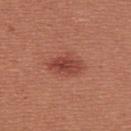The lesion was tiled from a total-body skin photograph and was not biopsied. The lesion-visualizer software estimated an area of roughly 7.5 mm² and a shape eccentricity near 0.8. And it measured a lesion color around L≈45 a*≈29 b*≈29 in CIELAB and a lesion-to-skin contrast of about 7.5 (normalized; higher = more distinct). It also reported a border-irregularity rating of about 2.5/10, a color-variation rating of about 4/10, and peripheral color asymmetry of about 1.5. And it measured a nevus-likeness score of about 95/100. On the upper back. The recorded lesion diameter is about 4 mm. The patient is a female in their mid- to late 20s. Imaged with white-light lighting. A lesion tile, about 15 mm wide, cut from a 3D total-body photograph.